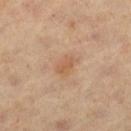Clinical impression: No biopsy was performed on this lesion — it was imaged during a full skin examination and was not determined to be concerning. Acquisition and patient details: The patient is a male aged around 60. The lesion is located on the left thigh. Measured at roughly 2.5 mm in maximum diameter. The tile uses cross-polarized illumination. A 15 mm crop from a total-body photograph taken for skin-cancer surveillance.